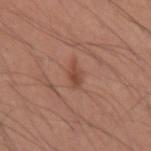workup: catalogued during a skin exam; not biopsied
lesion diameter: about 2.5 mm
anatomic site: the left upper arm
image: ~15 mm crop, total-body skin-cancer survey
tile lighting: white-light illumination
image-analysis metrics: a mean CIELAB color near L≈46 a*≈23 b*≈29 and about 9 CIELAB-L* units darker than the surrounding skin; a nevus-likeness score of about 55/100 and a lesion-detection confidence of about 100/100
subject: male, approximately 40 years of age